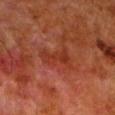Impression: Imaged during a routine full-body skin examination; the lesion was not biopsied and no histopathology is available. Context: On the left lower leg. Captured under cross-polarized illumination. A 15 mm close-up extracted from a 3D total-body photography capture. The total-body-photography lesion software estimated a footprint of about 8.5 mm², a shape eccentricity near 0.85, and a shape-asymmetry score of about 0.35 (0 = symmetric). It also reported an average lesion color of about L≈28 a*≈25 b*≈27 (CIELAB), about 5 CIELAB-L* units darker than the surrounding skin, and a lesion-to-skin contrast of about 6 (normalized; higher = more distinct). And it measured a nevus-likeness score of about 0/100 and a lesion-detection confidence of about 100/100. A male subject aged 78–82.